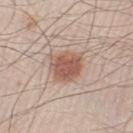No biopsy was performed on this lesion — it was imaged during a full skin examination and was not determined to be concerning. The lesion is located on the left lower leg. The patient is a male aged approximately 25. A 15 mm crop from a total-body photograph taken for skin-cancer surveillance. The lesion-visualizer software estimated an area of roughly 11 mm², an eccentricity of roughly 0.5, and a symmetry-axis asymmetry near 0.25. The tile uses white-light illumination.